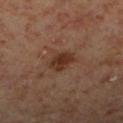Captured during whole-body skin photography for melanoma surveillance; the lesion was not biopsied. A 15 mm close-up tile from a total-body photography series done for melanoma screening. A male patient, aged 58–62. About 3 mm across. The lesion-visualizer software estimated a lesion area of about 6 mm² and an outline eccentricity of about 0.7 (0 = round, 1 = elongated). It also reported an average lesion color of about L≈30 a*≈18 b*≈25 (CIELAB), about 9 CIELAB-L* units darker than the surrounding skin, and a normalized lesion–skin contrast near 9. And it measured a classifier nevus-likeness of about 70/100. The lesion is on the right lower leg.